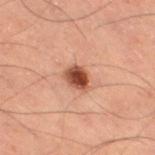{"biopsy_status": "not biopsied; imaged during a skin examination", "patient": {"sex": "male", "age_approx": 60}, "lighting": "cross-polarized", "site": "leg", "automated_metrics": {"area_mm2_approx": 5.0, "shape_asymmetry": 0.2, "cielab_L": 36, "cielab_a": 21, "cielab_b": 26, "vs_skin_darker_L": 15.0, "vs_skin_contrast_norm": 12.0, "border_irregularity_0_10": 1.5, "peripheral_color_asymmetry": 1.5}, "lesion_size": {"long_diameter_mm_approx": 2.5}, "image": {"source": "total-body photography crop", "field_of_view_mm": 15}}